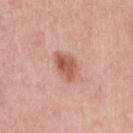Q: Is there a histopathology result?
A: total-body-photography surveillance lesion; no biopsy
Q: Lesion location?
A: the right upper arm
Q: What did automated image analysis measure?
A: a border-irregularity index near 2/10 and a color-variation rating of about 4/10; a nevus-likeness score of about 90/100 and lesion-presence confidence of about 100/100
Q: Patient demographics?
A: female, in their mid- to late 60s
Q: What kind of image is this?
A: ~15 mm tile from a whole-body skin photo